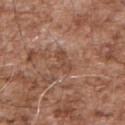Impression: Recorded during total-body skin imaging; not selected for excision or biopsy. Image and clinical context: A 15 mm close-up extracted from a 3D total-body photography capture. Located on the upper back. Automated image analysis of the tile measured an area of roughly 4.5 mm², a shape eccentricity near 0.6, and a symmetry-axis asymmetry near 0.6. It also reported a lesion color around L≈47 a*≈21 b*≈29 in CIELAB, a lesion–skin lightness drop of about 7, and a normalized lesion–skin contrast near 6. It also reported internal color variation of about 1.5 on a 0–10 scale. And it measured a classifier nevus-likeness of about 0/100 and lesion-presence confidence of about 100/100. A male subject, in their mid-50s. The recorded lesion diameter is about 3 mm.